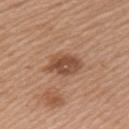Findings:
• workup: total-body-photography surveillance lesion; no biopsy
• imaging modality: ~15 mm crop, total-body skin-cancer survey
• illumination: white-light illumination
• subject: female, approximately 60 years of age
• lesion size: about 4 mm
• body site: the left upper arm